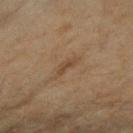follow-up: imaged on a skin check; not biopsied
TBP lesion metrics: a footprint of about 2 mm², a shape eccentricity near 0.95, and two-axis asymmetry of about 0.5; a lesion color around L≈40 a*≈15 b*≈30 in CIELAB, roughly 7 lightness units darker than nearby skin, and a lesion-to-skin contrast of about 6 (normalized; higher = more distinct); a nevus-likeness score of about 0/100 and lesion-presence confidence of about 95/100
lighting: cross-polarized
anatomic site: the right forearm
patient: female, in their 60s
diameter: ≈2.5 mm
image source: ~15 mm tile from a whole-body skin photo